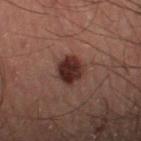Assessment: No biopsy was performed on this lesion — it was imaged during a full skin examination and was not determined to be concerning. Clinical summary: About 3.5 mm across. Cropped from a whole-body photographic skin survey; the tile spans about 15 mm. The subject is a male roughly 55 years of age. Imaged with cross-polarized lighting. Located on the left thigh. The total-body-photography lesion software estimated an area of roughly 8.5 mm² and a shape-asymmetry score of about 0.15 (0 = symmetric).